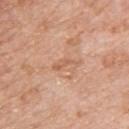{
  "lesion_size": {
    "long_diameter_mm_approx": 3.5
  },
  "site": "upper back",
  "image": {
    "source": "total-body photography crop",
    "field_of_view_mm": 15
  },
  "patient": {
    "sex": "female",
    "age_approx": 75
  }
}